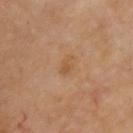Q: Is there a histopathology result?
A: no biopsy performed (imaged during a skin exam)
Q: Where on the body is the lesion?
A: the front of the torso
Q: What lighting was used for the tile?
A: cross-polarized
Q: What is the imaging modality?
A: ~15 mm tile from a whole-body skin photo
Q: Who is the patient?
A: male, about 55 years old
Q: Lesion size?
A: ~2.5 mm (longest diameter)
Q: What did automated image analysis measure?
A: a lesion color around L≈49 a*≈18 b*≈34 in CIELAB, roughly 6 lightness units darker than nearby skin, and a normalized lesion–skin contrast near 5.5; a classifier nevus-likeness of about 0/100 and a lesion-detection confidence of about 100/100